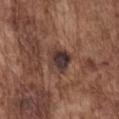This lesion was catalogued during total-body skin photography and was not selected for biopsy.
The patient is a male roughly 75 years of age.
Cropped from a total-body skin-imaging series; the visible field is about 15 mm.
Located on the chest.